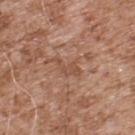Q: Was a biopsy performed?
A: no biopsy performed (imaged during a skin exam)
Q: Lesion location?
A: the upper back
Q: What kind of image is this?
A: ~15 mm crop, total-body skin-cancer survey
Q: Patient demographics?
A: male, about 55 years old
Q: What did automated image analysis measure?
A: an area of roughly 4 mm²; an average lesion color of about L≈50 a*≈21 b*≈30 (CIELAB) and about 6 CIELAB-L* units darker than the surrounding skin; an automated nevus-likeness rating near 0 out of 100
Q: What is the lesion's diameter?
A: about 3.5 mm